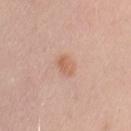• biopsy status: no biopsy performed (imaged during a skin exam)
• anatomic site: the arm
• lesion size: ≈2.5 mm
• illumination: white-light
• imaging modality: total-body-photography crop, ~15 mm field of view
• subject: male, aged around 45
• automated lesion analysis: a footprint of about 4.5 mm², an outline eccentricity of about 0.55 (0 = round, 1 = elongated), and a shape-asymmetry score of about 0.2 (0 = symmetric); a classifier nevus-likeness of about 70/100 and a detector confidence of about 100 out of 100 that the crop contains a lesion